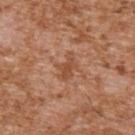Notes:
* follow-up · catalogued during a skin exam; not biopsied
* automated metrics · an average lesion color of about L≈50 a*≈24 b*≈33 (CIELAB), roughly 8 lightness units darker than nearby skin, and a normalized border contrast of about 6; border irregularity of about 5 on a 0–10 scale, internal color variation of about 2 on a 0–10 scale, and radial color variation of about 0.5; a detector confidence of about 100 out of 100 that the crop contains a lesion
* diameter · ≈3.5 mm
* location · the right upper arm
* image · total-body-photography crop, ~15 mm field of view
* tile lighting · white-light illumination
* subject · male, aged around 45